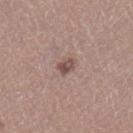Imaged during a routine full-body skin examination; the lesion was not biopsied and no histopathology is available. A region of skin cropped from a whole-body photographic capture, roughly 15 mm wide. The patient is a female aged 33 to 37. On the left leg.